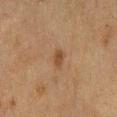Case summary:
- follow-up · no biopsy performed (imaged during a skin exam)
- lesion diameter · about 2.5 mm
- TBP lesion metrics · a border-irregularity index near 3/10 and a color-variation rating of about 0.5/10; a nevus-likeness score of about 25/100
- image source · ~15 mm crop, total-body skin-cancer survey
- anatomic site · the chest
- patient · male, about 75 years old